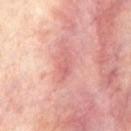This lesion was catalogued during total-body skin photography and was not selected for biopsy.
From the chest.
Approximately 4 mm at its widest.
A roughly 15 mm field-of-view crop from a total-body skin photograph.
Imaged with cross-polarized lighting.
A male subject, in their mid-30s.
The total-body-photography lesion software estimated a footprint of about 5 mm² and a symmetry-axis asymmetry near 0.4. It also reported a classifier nevus-likeness of about 0/100 and lesion-presence confidence of about 100/100.